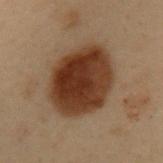Assessment: The lesion was photographed on a routine skin check and not biopsied; there is no pathology result. Acquisition and patient details: A 15 mm crop from a total-body photograph taken for skin-cancer surveillance. Located on the upper back. A male subject, roughly 55 years of age. This is a cross-polarized tile.